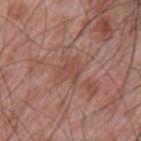Captured during whole-body skin photography for melanoma surveillance; the lesion was not biopsied.
The lesion is located on the left upper arm.
A 15 mm close-up tile from a total-body photography series done for melanoma screening.
A male subject approximately 65 years of age.